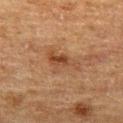This lesion was catalogued during total-body skin photography and was not selected for biopsy.
A lesion tile, about 15 mm wide, cut from a 3D total-body photograph.
The lesion is on the left thigh.
The lesion's longest dimension is about 4.5 mm.
The patient is a male approximately 75 years of age.
This is a cross-polarized tile.